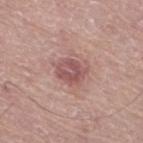biopsy status = no biopsy performed (imaged during a skin exam) | image source = total-body-photography crop, ~15 mm field of view | size = about 3.5 mm | image-analysis metrics = an automated nevus-likeness rating near 0 out of 100 | site = the leg | subject = male, about 75 years old.